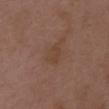Clinical impression: The lesion was photographed on a routine skin check and not biopsied; there is no pathology result. Background: The lesion is located on the upper back. A female subject, aged around 30. An algorithmic analysis of the crop reported an average lesion color of about L≈41 a*≈18 b*≈27 (CIELAB) and about 4 CIELAB-L* units darker than the surrounding skin. This image is a 15 mm lesion crop taken from a total-body photograph. Measured at roughly 2.5 mm in maximum diameter.